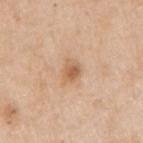This lesion was catalogued during total-body skin photography and was not selected for biopsy.
A male patient, aged 58–62.
Cropped from a whole-body photographic skin survey; the tile spans about 15 mm.
Located on the arm.